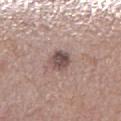Clinical impression: The lesion was tiled from a total-body skin photograph and was not biopsied. Acquisition and patient details: A close-up tile cropped from a whole-body skin photograph, about 15 mm across. A male subject in their mid- to late 50s. Imaged with white-light lighting. The lesion is on the right lower leg. Automated tile analysis of the lesion measured border irregularity of about 1.5 on a 0–10 scale and a color-variation rating of about 4.5/10. And it measured an automated nevus-likeness rating near 30 out of 100 and a detector confidence of about 100 out of 100 that the crop contains a lesion. The lesion's longest dimension is about 3 mm.